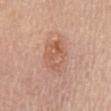The lesion was tiled from a total-body skin photograph and was not biopsied. From the chest. A female subject about 65 years old. A close-up tile cropped from a whole-body skin photograph, about 15 mm across.